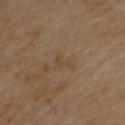Imaged during a routine full-body skin examination; the lesion was not biopsied and no histopathology is available. On the upper back. A roughly 15 mm field-of-view crop from a total-body skin photograph. Measured at roughly 3 mm in maximum diameter. The tile uses cross-polarized illumination. A male patient, in their mid- to late 50s. An algorithmic analysis of the crop reported a lesion–skin lightness drop of about 5. And it measured a nevus-likeness score of about 0/100 and lesion-presence confidence of about 100/100.